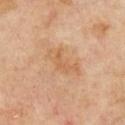Clinical summary: The tile uses cross-polarized illumination. Measured at roughly 5 mm in maximum diameter. The lesion is located on the chest. A lesion tile, about 15 mm wide, cut from a 3D total-body photograph. A male patient, about 70 years old. Automated image analysis of the tile measured a lesion area of about 8 mm². And it measured a mean CIELAB color near L≈60 a*≈20 b*≈36, roughly 7 lightness units darker than nearby skin, and a normalized lesion–skin contrast near 5. And it measured border irregularity of about 5 on a 0–10 scale, a within-lesion color-variation index near 2.5/10, and peripheral color asymmetry of about 1.